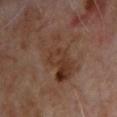{"biopsy_status": "not biopsied; imaged during a skin examination", "site": "chest", "image": {"source": "total-body photography crop", "field_of_view_mm": 15}, "lighting": "cross-polarized", "lesion_size": {"long_diameter_mm_approx": 7.0}, "automated_metrics": {"cielab_L": 34, "cielab_a": 17, "cielab_b": 26, "vs_skin_darker_L": 7.0, "vs_skin_contrast_norm": 7.0, "nevus_likeness_0_100": 5}, "patient": {"sex": "male", "age_approx": 65}}